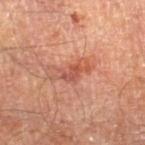Notes:
* notes — total-body-photography surveillance lesion; no biopsy